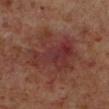follow-up — no biopsy performed (imaged during a skin exam)
body site — the left lower leg
subject — male, roughly 60 years of age
automated lesion analysis — a mean CIELAB color near L≈31 a*≈23 b*≈21, about 6 CIELAB-L* units darker than the surrounding skin, and a lesion-to-skin contrast of about 7 (normalized; higher = more distinct); a nevus-likeness score of about 0/100 and a lesion-detection confidence of about 100/100
acquisition — ~15 mm tile from a whole-body skin photo
lesion size — ≈6.5 mm
tile lighting — cross-polarized